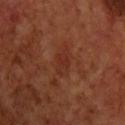Clinical impression: This lesion was catalogued during total-body skin photography and was not selected for biopsy. Context: The tile uses cross-polarized illumination. From the front of the torso. Automated image analysis of the tile measured an eccentricity of roughly 0.8 and a symmetry-axis asymmetry near 0.35. The analysis additionally found a lesion color around L≈29 a*≈25 b*≈28 in CIELAB and a lesion–skin lightness drop of about 5. The analysis additionally found a color-variation rating of about 2/10 and a peripheral color-asymmetry measure near 1. About 2.5 mm across. A male subject about 70 years old. A region of skin cropped from a whole-body photographic capture, roughly 15 mm wide.